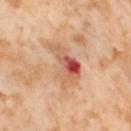Captured during whole-body skin photography for melanoma surveillance; the lesion was not biopsied.
A female subject, aged 53–57.
Captured under cross-polarized illumination.
Automated image analysis of the tile measured a mean CIELAB color near L≈60 a*≈26 b*≈34, about 12 CIELAB-L* units darker than the surrounding skin, and a normalized lesion–skin contrast near 7.5. And it measured border irregularity of about 5.5 on a 0–10 scale, internal color variation of about 10 on a 0–10 scale, and radial color variation of about 5. The software also gave an automated nevus-likeness rating near 0 out of 100 and lesion-presence confidence of about 100/100.
The recorded lesion diameter is about 5.5 mm.
The lesion is on the left thigh.
This image is a 15 mm lesion crop taken from a total-body photograph.